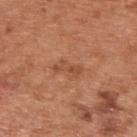Findings:
– subject — male, in their mid- to late 60s
– image source — ~15 mm crop, total-body skin-cancer survey
– site — the upper back
– size — ~3.5 mm (longest diameter)
– illumination — white-light illumination